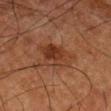| feature | finding |
|---|---|
| follow-up | imaged on a skin check; not biopsied |